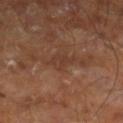The lesion was photographed on a routine skin check and not biopsied; there is no pathology result.
From the right leg.
Cropped from a total-body skin-imaging series; the visible field is about 15 mm.
The subject is a male roughly 60 years of age.
The tile uses cross-polarized illumination.